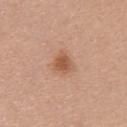Captured during whole-body skin photography for melanoma surveillance; the lesion was not biopsied.
Cropped from a total-body skin-imaging series; the visible field is about 15 mm.
Automated image analysis of the tile measured a shape eccentricity near 0.5 and two-axis asymmetry of about 0.3. It also reported a mean CIELAB color near L≈55 a*≈23 b*≈33 and a lesion–skin lightness drop of about 10. It also reported a detector confidence of about 100 out of 100 that the crop contains a lesion.
The lesion is on the upper back.
The patient is a female about 40 years old.